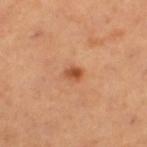<lesion>
<biopsy_status>not biopsied; imaged during a skin examination</biopsy_status>
<patient>
  <sex>female</sex>
  <age_approx>55</age_approx>
</patient>
<image>
  <source>total-body photography crop</source>
  <field_of_view_mm>15</field_of_view_mm>
</image>
<site>left thigh</site>
</lesion>